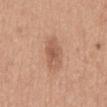<case>
<biopsy_status>not biopsied; imaged during a skin examination</biopsy_status>
<lighting>white-light</lighting>
<site>mid back</site>
<lesion_size>
  <long_diameter_mm_approx>5.0</long_diameter_mm_approx>
</lesion_size>
<automated_metrics>
  <border_irregularity_0_10>3.0</border_irregularity_0_10>
  <color_variation_0_10>3.5</color_variation_0_10>
  <peripheral_color_asymmetry>1.0</peripheral_color_asymmetry>
  <nevus_likeness_0_100>20</nevus_likeness_0_100>
  <lesion_detection_confidence_0_100>100</lesion_detection_confidence_0_100>
</automated_metrics>
<image>
  <source>total-body photography crop</source>
  <field_of_view_mm>15</field_of_view_mm>
</image>
<patient>
  <sex>male</sex>
  <age_approx>30</age_approx>
</patient>
</case>